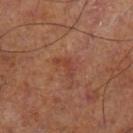biopsy status: catalogued during a skin exam; not biopsied
subject: male, about 70 years old
acquisition: ~15 mm crop, total-body skin-cancer survey
site: the left lower leg